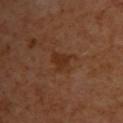Assessment:
Part of a total-body skin-imaging series; this lesion was reviewed on a skin check and was not flagged for biopsy.
Background:
The subject is a female roughly 40 years of age. Captured under cross-polarized illumination. A region of skin cropped from a whole-body photographic capture, roughly 15 mm wide. Approximately 3 mm at its widest. On the upper back.